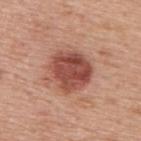workup = imaged on a skin check; not biopsied | size = ~5 mm (longest diameter) | lighting = white-light | anatomic site = the upper back | subject = male, about 75 years old | TBP lesion metrics = an area of roughly 17 mm², an eccentricity of roughly 0.4, and two-axis asymmetry of about 0.15; a lesion color around L≈49 a*≈27 b*≈28 in CIELAB, roughly 14 lightness units darker than nearby skin, and a normalized border contrast of about 9.5; a border-irregularity rating of about 2/10, a color-variation rating of about 5/10, and radial color variation of about 1.5; an automated nevus-likeness rating near 95 out of 100 and a detector confidence of about 100 out of 100 that the crop contains a lesion | acquisition = ~15 mm tile from a whole-body skin photo.